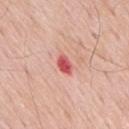Recorded during total-body skin imaging; not selected for excision or biopsy. The patient is a male aged around 65. This image is a 15 mm lesion crop taken from a total-body photograph. Located on the mid back.